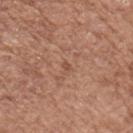notes: total-body-photography surveillance lesion; no biopsy | body site: the left upper arm | lighting: white-light | size: ≈1 mm | patient: female, aged approximately 75 | image source: 15 mm crop, total-body photography | automated metrics: an area of roughly 1 mm² and an eccentricity of roughly 0.75; radial color variation of about 0; an automated nevus-likeness rating near 0 out of 100 and a lesion-detection confidence of about 100/100.